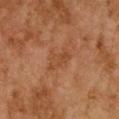lighting: cross-polarized illumination
image source: ~15 mm tile from a whole-body skin photo
patient: female, aged around 60
anatomic site: the chest